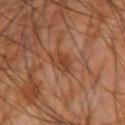Clinical impression:
No biopsy was performed on this lesion — it was imaged during a full skin examination and was not determined to be concerning.
Context:
A 15 mm crop from a total-body photograph taken for skin-cancer surveillance. This is a cross-polarized tile. The lesion's longest dimension is about 3.5 mm. A male subject approximately 65 years of age. From the right forearm.